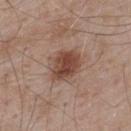Findings:
– follow-up · catalogued during a skin exam; not biopsied
– acquisition · 15 mm crop, total-body photography
– tile lighting · white-light illumination
– anatomic site · the upper back
– automated metrics · two-axis asymmetry of about 0.2; an average lesion color of about L≈46 a*≈20 b*≈26 (CIELAB), about 12 CIELAB-L* units darker than the surrounding skin, and a normalized border contrast of about 9; a border-irregularity rating of about 2.5/10 and radial color variation of about 1.5; an automated nevus-likeness rating near 95 out of 100 and lesion-presence confidence of about 100/100
– patient · male, about 55 years old